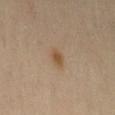Q: What lighting was used for the tile?
A: cross-polarized illumination
Q: What kind of image is this?
A: total-body-photography crop, ~15 mm field of view
Q: Who is the patient?
A: female, aged 43 to 47
Q: Where on the body is the lesion?
A: the left thigh
Q: Lesion size?
A: ~2.5 mm (longest diameter)